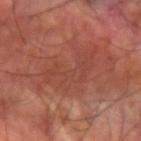{
  "biopsy_status": "not biopsied; imaged during a skin examination",
  "automated_metrics": {
    "border_irregularity_0_10": 8.5,
    "color_variation_0_10": 3.0,
    "peripheral_color_asymmetry": 1.0
  },
  "lesion_size": {
    "long_diameter_mm_approx": 8.5
  },
  "site": "left forearm",
  "lighting": "cross-polarized",
  "image": {
    "source": "total-body photography crop",
    "field_of_view_mm": 15
  },
  "patient": {
    "sex": "male",
    "age_approx": 60
  }
}